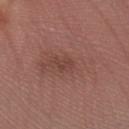Case summary:
– patient — female, roughly 55 years of age
– image source — ~15 mm tile from a whole-body skin photo
– body site — the right forearm